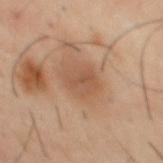| feature | finding |
|---|---|
| workup | imaged on a skin check; not biopsied |
| subject | male, aged approximately 40 |
| image source | total-body-photography crop, ~15 mm field of view |
| lesion size | about 3 mm |
| body site | the mid back |
| lighting | cross-polarized |